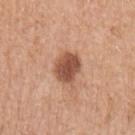Q: Is there a histopathology result?
A: no biopsy performed (imaged during a skin exam)
Q: Lesion location?
A: the left upper arm
Q: How was this image acquired?
A: ~15 mm tile from a whole-body skin photo
Q: Automated lesion metrics?
A: a lesion area of about 9 mm², a shape eccentricity near 0.6, and a symmetry-axis asymmetry near 0.15; an average lesion color of about L≈52 a*≈23 b*≈31 (CIELAB), roughly 15 lightness units darker than nearby skin, and a lesion-to-skin contrast of about 10 (normalized; higher = more distinct); a within-lesion color-variation index near 3.5/10 and radial color variation of about 1; an automated nevus-likeness rating near 80 out of 100 and a lesion-detection confidence of about 100/100
Q: Patient demographics?
A: female, about 70 years old
Q: Lesion size?
A: ≈3.5 mm